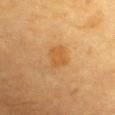No biopsy was performed on this lesion — it was imaged during a full skin examination and was not determined to be concerning.
The recorded lesion diameter is about 2.5 mm.
A close-up tile cropped from a whole-body skin photograph, about 15 mm across.
Imaged with cross-polarized lighting.
The patient is a male aged 83 or over.
From the chest.